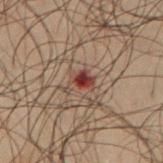The lesion was tiled from a total-body skin photograph and was not biopsied.
Captured under cross-polarized illumination.
Located on the arm.
Approximately 3 mm at its widest.
A lesion tile, about 15 mm wide, cut from a 3D total-body photograph.
A male patient, about 50 years old.